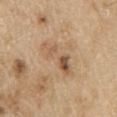Clinical impression: Part of a total-body skin-imaging series; this lesion was reviewed on a skin check and was not flagged for biopsy. Context: The patient is a male approximately 70 years of age. Captured under white-light illumination. A 15 mm crop from a total-body photograph taken for skin-cancer surveillance. Approximately 6 mm at its widest. The lesion is located on the left upper arm.